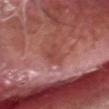Impression:
Recorded during total-body skin imaging; not selected for excision or biopsy.
Context:
The lesion's longest dimension is about 4 mm. The total-body-photography lesion software estimated an average lesion color of about L≈46 a*≈29 b*≈26 (CIELAB) and about 7 CIELAB-L* units darker than the surrounding skin. The software also gave a border-irregularity rating of about 6.5/10, a color-variation rating of about 1/10, and radial color variation of about 0.5. A 15 mm close-up tile from a total-body photography series done for melanoma screening. The lesion is located on the head or neck. The patient is a male aged around 65.